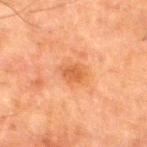Part of a total-body skin-imaging series; this lesion was reviewed on a skin check and was not flagged for biopsy. The lesion is on the right thigh. Approximately 2.5 mm at its widest. A male subject roughly 80 years of age. A 15 mm close-up tile from a total-body photography series done for melanoma screening.